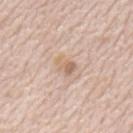Clinical impression: Captured during whole-body skin photography for melanoma surveillance; the lesion was not biopsied. Acquisition and patient details: Automated image analysis of the tile measured an eccentricity of roughly 0.7 and a symmetry-axis asymmetry near 0.3. It also reported a mean CIELAB color near L≈65 a*≈16 b*≈30, roughly 8 lightness units darker than nearby skin, and a normalized lesion–skin contrast near 6. It also reported an automated nevus-likeness rating near 5 out of 100 and lesion-presence confidence of about 100/100. A roughly 15 mm field-of-view crop from a total-body skin photograph. Measured at roughly 2.5 mm in maximum diameter. A male subject approximately 80 years of age. Imaged with white-light lighting. On the mid back.